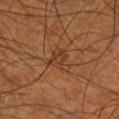Q: Is there a histopathology result?
A: imaged on a skin check; not biopsied
Q: How was the tile lit?
A: cross-polarized illumination
Q: Lesion location?
A: the right lower leg
Q: What is the imaging modality?
A: 15 mm crop, total-body photography
Q: Lesion size?
A: ≈3.5 mm
Q: Who is the patient?
A: male, aged 68–72
Q: Automated lesion metrics?
A: a footprint of about 6 mm² and two-axis asymmetry of about 0.5; a nevus-likeness score of about 0/100 and a detector confidence of about 85 out of 100 that the crop contains a lesion